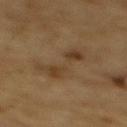<record>
<biopsy_status>not biopsied; imaged during a skin examination</biopsy_status>
<site>back</site>
<patient>
  <sex>male</sex>
  <age_approx>85+</age_approx>
</patient>
<lighting>cross-polarized</lighting>
<image>
  <source>total-body photography crop</source>
  <field_of_view_mm>15</field_of_view_mm>
</image>
</record>